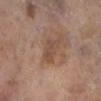Assessment:
Recorded during total-body skin imaging; not selected for excision or biopsy.
Background:
On the leg. A female subject approximately 85 years of age or older. A region of skin cropped from a whole-body photographic capture, roughly 15 mm wide. Captured under white-light illumination. Approximately 3.5 mm at its widest.